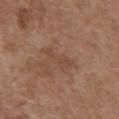Clinical impression:
The lesion was photographed on a routine skin check and not biopsied; there is no pathology result.
Image and clinical context:
About 4 mm across. This is a white-light tile. A male subject aged 73–77. The lesion is located on the front of the torso. Cropped from a whole-body photographic skin survey; the tile spans about 15 mm.